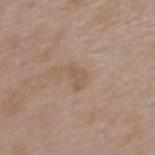  biopsy_status: not biopsied; imaged during a skin examination
  lesion_size:
    long_diameter_mm_approx: 2.5
  automated_metrics:
    area_mm2_approx: 3.0
    eccentricity: 0.8
    shape_asymmetry: 0.45
    nevus_likeness_0_100: 0
  lighting: white-light
  patient:
    sex: female
    age_approx: 40
  site: upper back
  image:
    source: total-body photography crop
    field_of_view_mm: 15